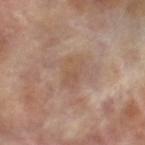No biopsy was performed on this lesion — it was imaged during a full skin examination and was not determined to be concerning. Approximately 3.5 mm at its widest. Captured under cross-polarized illumination. A 15 mm crop from a total-body photograph taken for skin-cancer surveillance. The lesion is on the right forearm. An algorithmic analysis of the crop reported a lesion area of about 7 mm², an eccentricity of roughly 0.8, and a symmetry-axis asymmetry near 0.2. The analysis additionally found a lesion color around L≈53 a*≈16 b*≈28 in CIELAB, about 6 CIELAB-L* units darker than the surrounding skin, and a normalized border contrast of about 4.5. The analysis additionally found a border-irregularity index near 2.5/10, a color-variation rating of about 3.5/10, and radial color variation of about 1. A female subject in their mid- to late 70s.